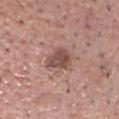Assessment:
The lesion was tiled from a total-body skin photograph and was not biopsied.
Context:
From the head or neck. Imaged with white-light lighting. The recorded lesion diameter is about 3.5 mm. The total-body-photography lesion software estimated a mean CIELAB color near L≈49 a*≈21 b*≈23. The software also gave a nevus-likeness score of about 20/100. A male subject aged 38 to 42. A 15 mm close-up tile from a total-body photography series done for melanoma screening.